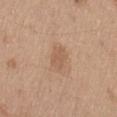Impression: The lesion was photographed on a routine skin check and not biopsied; there is no pathology result. Clinical summary: Approximately 3 mm at its widest. The lesion is located on the lower back. This is a white-light tile. A lesion tile, about 15 mm wide, cut from a 3D total-body photograph. The lesion-visualizer software estimated a footprint of about 4.5 mm², an outline eccentricity of about 0.75 (0 = round, 1 = elongated), and a shape-asymmetry score of about 0.25 (0 = symmetric). The software also gave an average lesion color of about L≈57 a*≈19 b*≈32 (CIELAB). The software also gave internal color variation of about 1.5 on a 0–10 scale. A male subject about 70 years old.